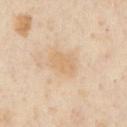Automated image analysis of the tile measured an area of roughly 6.5 mm², an outline eccentricity of about 0.75 (0 = round, 1 = elongated), and a shape-asymmetry score of about 0.2 (0 = symmetric). The analysis additionally found an automated nevus-likeness rating near 0 out of 100 and a lesion-detection confidence of about 100/100. The subject is a male aged around 55. The recorded lesion diameter is about 3.5 mm. Cropped from a whole-body photographic skin survey; the tile spans about 15 mm. The lesion is located on the front of the torso.